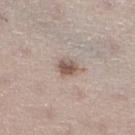follow-up — total-body-photography surveillance lesion; no biopsy
TBP lesion metrics — an outline eccentricity of about 0.6 (0 = round, 1 = elongated); a mean CIELAB color near L≈55 a*≈16 b*≈23, a lesion–skin lightness drop of about 12, and a normalized border contrast of about 8.5; a color-variation rating of about 4.5/10 and radial color variation of about 1.5
lighting — white-light illumination
anatomic site — the right lower leg
patient — female, aged around 50
lesion diameter — ~2.5 mm (longest diameter)
imaging modality — ~15 mm tile from a whole-body skin photo